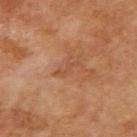notes = imaged on a skin check; not biopsied | subject = male, approximately 70 years of age | lesion size = about 3 mm | body site = the right upper arm | TBP lesion metrics = an average lesion color of about L≈49 a*≈23 b*≈34 (CIELAB), roughly 6 lightness units darker than nearby skin, and a normalized lesion–skin contrast near 4.5; a border-irregularity index near 6.5/10 and internal color variation of about 0 on a 0–10 scale | image = total-body-photography crop, ~15 mm field of view | illumination = cross-polarized.